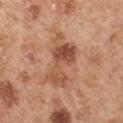Impression:
Recorded during total-body skin imaging; not selected for excision or biopsy.
Image and clinical context:
Automated tile analysis of the lesion measured a border-irregularity rating of about 7/10 and internal color variation of about 6.5 on a 0–10 scale. The patient is a male aged 53–57. This is a white-light tile. Located on the left upper arm. Longest diameter approximately 6.5 mm. A lesion tile, about 15 mm wide, cut from a 3D total-body photograph.